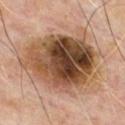Notes:
– workup: no biopsy performed (imaged during a skin exam)
– location: the chest
– subject: male, roughly 65 years of age
– lesion diameter: ~9.5 mm (longest diameter)
– illumination: cross-polarized
– acquisition: ~15 mm tile from a whole-body skin photo